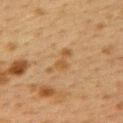Q: Was this lesion biopsied?
A: total-body-photography surveillance lesion; no biopsy
Q: Automated lesion metrics?
A: a mean CIELAB color near L≈45 a*≈16 b*≈34, a lesion–skin lightness drop of about 6, and a lesion-to-skin contrast of about 6 (normalized; higher = more distinct); border irregularity of about 7 on a 0–10 scale, a within-lesion color-variation index near 1/10, and peripheral color asymmetry of about 0
Q: How was this image acquired?
A: total-body-photography crop, ~15 mm field of view
Q: Patient demographics?
A: female, aged 38 to 42
Q: Illumination type?
A: cross-polarized
Q: What is the anatomic site?
A: the upper back
Q: Lesion size?
A: ~4 mm (longest diameter)